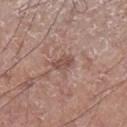Notes:
- notes · imaged on a skin check; not biopsied
- patient · male, aged 58 to 62
- body site · the left lower leg
- acquisition · total-body-photography crop, ~15 mm field of view